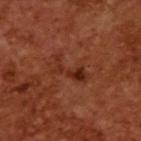Clinical impression:
Part of a total-body skin-imaging series; this lesion was reviewed on a skin check and was not flagged for biopsy.